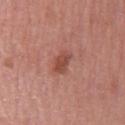Context:
A lesion tile, about 15 mm wide, cut from a 3D total-body photograph. A female patient, about 70 years old. The lesion is located on the front of the torso. About 3 mm across.